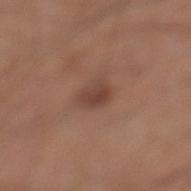| feature | finding |
|---|---|
| notes | imaged on a skin check; not biopsied |
| image | 15 mm crop, total-body photography |
| subject | male, in their 30s |
| body site | the left lower leg |